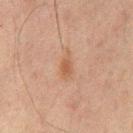Q: Was a biopsy performed?
A: imaged on a skin check; not biopsied
Q: What lighting was used for the tile?
A: cross-polarized
Q: Lesion location?
A: the upper back
Q: What did automated image analysis measure?
A: a mean CIELAB color near L≈46 a*≈17 b*≈28, roughly 6 lightness units darker than nearby skin, and a lesion-to-skin contrast of about 6 (normalized; higher = more distinct); a border-irregularity index near 3/10, a within-lesion color-variation index near 2/10, and a peripheral color-asymmetry measure near 0.5; a classifier nevus-likeness of about 10/100
Q: What is the lesion's diameter?
A: about 3.5 mm
Q: What kind of image is this?
A: 15 mm crop, total-body photography
Q: What are the patient's age and sex?
A: male, aged 63 to 67